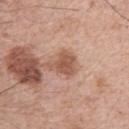The lesion was photographed on a routine skin check and not biopsied; there is no pathology result.
A 15 mm close-up tile from a total-body photography series done for melanoma screening.
The total-body-photography lesion software estimated a lesion area of about 6 mm², a shape eccentricity near 0.4, and a shape-asymmetry score of about 0.35 (0 = symmetric). The analysis additionally found a lesion color around L≈54 a*≈22 b*≈30 in CIELAB, a lesion–skin lightness drop of about 11, and a normalized border contrast of about 8. And it measured a nevus-likeness score of about 30/100 and lesion-presence confidence of about 100/100.
This is a white-light tile.
The patient is a male in their mid-60s.
Longest diameter approximately 3 mm.
On the right upper arm.